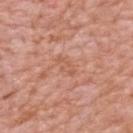• notes — no biopsy performed (imaged during a skin exam)
• site — the upper back
• tile lighting — white-light illumination
• lesion diameter — ~3 mm (longest diameter)
• patient — female, roughly 70 years of age
• automated metrics — a footprint of about 3 mm² and a shape-asymmetry score of about 0.2 (0 = symmetric); a border-irregularity index near 3.5/10 and a peripheral color-asymmetry measure near 0; an automated nevus-likeness rating near 0 out of 100 and a lesion-detection confidence of about 100/100
• acquisition — ~15 mm tile from a whole-body skin photo